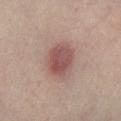Part of a total-body skin-imaging series; this lesion was reviewed on a skin check and was not flagged for biopsy. On the left lower leg. A close-up tile cropped from a whole-body skin photograph, about 15 mm across. The lesion-visualizer software estimated a lesion color around L≈47 a*≈21 b*≈20 in CIELAB, a lesion–skin lightness drop of about 11, and a normalized border contrast of about 8. It also reported a border-irregularity rating of about 1/10 and internal color variation of about 3.5 on a 0–10 scale. This is a cross-polarized tile. The subject is a male aged around 65.